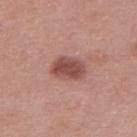workup: no biopsy performed (imaged during a skin exam)
body site: the upper back
imaging modality: ~15 mm crop, total-body skin-cancer survey
patient: female, aged around 50
illumination: white-light
automated metrics: about 13 CIELAB-L* units darker than the surrounding skin and a lesion-to-skin contrast of about 9 (normalized; higher = more distinct); a detector confidence of about 100 out of 100 that the crop contains a lesion